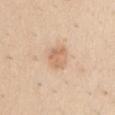<lesion>
  <biopsy_status>not biopsied; imaged during a skin examination</biopsy_status>
  <patient>
    <sex>male</sex>
    <age_approx>40</age_approx>
  </patient>
  <lesion_size>
    <long_diameter_mm_approx>3.0</long_diameter_mm_approx>
  </lesion_size>
  <image>
    <source>total-body photography crop</source>
    <field_of_view_mm>15</field_of_view_mm>
  </image>
  <site>chest</site>
</lesion>